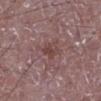notes: catalogued during a skin exam; not biopsied.